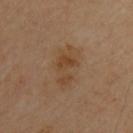Q: Was this lesion biopsied?
A: imaged on a skin check; not biopsied
Q: Where on the body is the lesion?
A: the upper back
Q: What did automated image analysis measure?
A: an outline eccentricity of about 0.85 (0 = round, 1 = elongated) and a symmetry-axis asymmetry near 0.35; a mean CIELAB color near L≈45 a*≈17 b*≈32, roughly 6 lightness units darker than nearby skin, and a normalized border contrast of about 6; a border-irregularity rating of about 4.5/10, a within-lesion color-variation index near 4/10, and a peripheral color-asymmetry measure near 1.5
Q: Patient demographics?
A: male, in their mid-50s
Q: How was the tile lit?
A: cross-polarized illumination
Q: What is the imaging modality?
A: 15 mm crop, total-body photography